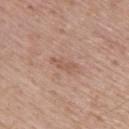notes: no biopsy performed (imaged during a skin exam)
tile lighting: white-light
body site: the upper back
image: ~15 mm tile from a whole-body skin photo
subject: male, aged approximately 75
lesion diameter: ~3 mm (longest diameter)